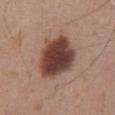Recorded during total-body skin imaging; not selected for excision or biopsy. A male subject, in their mid- to late 60s. The total-body-photography lesion software estimated a lesion color around L≈41 a*≈20 b*≈24 in CIELAB and a normalized lesion–skin contrast near 13.5. And it measured a border-irregularity index near 2/10, a color-variation rating of about 5/10, and peripheral color asymmetry of about 1.5. The software also gave a nevus-likeness score of about 80/100 and a lesion-detection confidence of about 100/100. About 6 mm across. A 15 mm close-up tile from a total-body photography series done for melanoma screening. The lesion is on the abdomen.